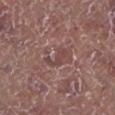Findings:
• notes — imaged on a skin check; not biopsied
• patient — male, in their 80s
• size — about 3.5 mm
• image source — 15 mm crop, total-body photography
• location — the left lower leg
• illumination — white-light